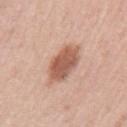Clinical impression: Imaged during a routine full-body skin examination; the lesion was not biopsied and no histopathology is available. Clinical summary: A male patient approximately 55 years of age. A close-up tile cropped from a whole-body skin photograph, about 15 mm across. About 5 mm across. Captured under white-light illumination. The lesion-visualizer software estimated a footprint of about 13 mm². And it measured a border-irregularity rating of about 2/10, internal color variation of about 3.5 on a 0–10 scale, and peripheral color asymmetry of about 1. On the left upper arm.